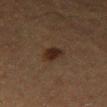Part of a total-body skin-imaging series; this lesion was reviewed on a skin check and was not flagged for biopsy.
Approximately 3 mm at its widest.
Cropped from a total-body skin-imaging series; the visible field is about 15 mm.
The lesion is located on the left thigh.
A male patient, aged 73 to 77.
The tile uses cross-polarized illumination.
Automated image analysis of the tile measured a lesion area of about 4.5 mm², a shape eccentricity near 0.7, and a symmetry-axis asymmetry near 0.2.